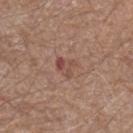This lesion was catalogued during total-body skin photography and was not selected for biopsy. On the left forearm. Automated tile analysis of the lesion measured a lesion area of about 4.5 mm² and two-axis asymmetry of about 0.45. And it measured a lesion color around L≈48 a*≈21 b*≈25 in CIELAB and roughly 8 lightness units darker than nearby skin. The software also gave a color-variation rating of about 8.5/10 and peripheral color asymmetry of about 2.5. Cropped from a whole-body photographic skin survey; the tile spans about 15 mm. Imaged with white-light lighting. The patient is a male aged 73 to 77.